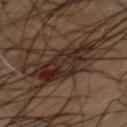Imaged during a routine full-body skin examination; the lesion was not biopsied and no histopathology is available. The patient is a male in their 50s. The recorded lesion diameter is about 6 mm. Captured under cross-polarized illumination. Located on the mid back. A lesion tile, about 15 mm wide, cut from a 3D total-body photograph.